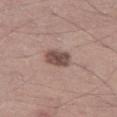notes — catalogued during a skin exam; not biopsied | imaging modality — 15 mm crop, total-body photography | location — the left thigh | patient — male, approximately 45 years of age | TBP lesion metrics — a lesion area of about 6 mm², an outline eccentricity of about 0.7 (0 = round, 1 = elongated), and two-axis asymmetry of about 0.2; a lesion color around L≈48 a*≈17 b*≈22 in CIELAB and a normalized border contrast of about 10; a border-irregularity rating of about 2/10, internal color variation of about 2.5 on a 0–10 scale, and a peripheral color-asymmetry measure near 0.5.